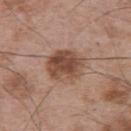<tbp_lesion>
  <biopsy_status>not biopsied; imaged during a skin examination</biopsy_status>
  <image>
    <source>total-body photography crop</source>
    <field_of_view_mm>15</field_of_view_mm>
  </image>
  <lesion_size>
    <long_diameter_mm_approx>4.5</long_diameter_mm_approx>
  </lesion_size>
  <site>upper back</site>
  <patient>
    <sex>male</sex>
    <age_approx>65</age_approx>
  </patient>
</tbp_lesion>